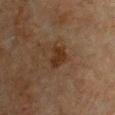Captured during whole-body skin photography for melanoma surveillance; the lesion was not biopsied. The patient is a male in their mid- to late 60s. Approximately 3 mm at its widest. A close-up tile cropped from a whole-body skin photograph, about 15 mm across. The lesion is located on the front of the torso. Imaged with cross-polarized lighting.